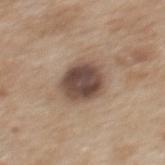Impression: Part of a total-body skin-imaging series; this lesion was reviewed on a skin check and was not flagged for biopsy. Image and clinical context: The patient is a female aged around 40. Cropped from a total-body skin-imaging series; the visible field is about 15 mm. On the mid back. Imaged with white-light lighting.